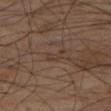follow-up: catalogued during a skin exam; not biopsied
patient: male, aged approximately 55
image: total-body-photography crop, ~15 mm field of view
location: the leg
illumination: cross-polarized
automated metrics: an area of roughly 3 mm², a shape eccentricity near 0.85, and a shape-asymmetry score of about 0.5 (0 = symmetric); a classifier nevus-likeness of about 0/100 and lesion-presence confidence of about 95/100